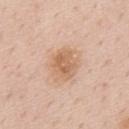follow-up=imaged on a skin check; not biopsied
site=the mid back
patient=male, approximately 60 years of age
acquisition=~15 mm tile from a whole-body skin photo